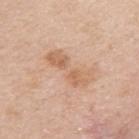workup = imaged on a skin check; not biopsied
anatomic site = the right upper arm
size = ≈6 mm
subject = female, approximately 45 years of age
TBP lesion metrics = a lesion area of about 11 mm² and a shape eccentricity near 0.95
acquisition = ~15 mm tile from a whole-body skin photo
illumination = white-light